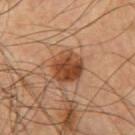{"biopsy_status": "not biopsied; imaged during a skin examination", "image": {"source": "total-body photography crop", "field_of_view_mm": 15}, "patient": {"sex": "male", "age_approx": 60}, "site": "right upper arm"}